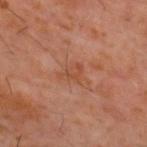Q: Was this lesion biopsied?
A: catalogued during a skin exam; not biopsied
Q: Who is the patient?
A: male, aged 58–62
Q: Automated lesion metrics?
A: a lesion area of about 3.5 mm² and a shape eccentricity near 0.7; an average lesion color of about L≈45 a*≈24 b*≈31 (CIELAB), about 6 CIELAB-L* units darker than the surrounding skin, and a lesion-to-skin contrast of about 5.5 (normalized; higher = more distinct); a border-irregularity rating of about 5.5/10, a within-lesion color-variation index near 1/10, and radial color variation of about 0.5; a classifier nevus-likeness of about 0/100
Q: What is the lesion's diameter?
A: ~2.5 mm (longest diameter)
Q: What is the imaging modality?
A: ~15 mm tile from a whole-body skin photo
Q: What lighting was used for the tile?
A: cross-polarized illumination
Q: What is the anatomic site?
A: the mid back